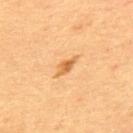Recorded during total-body skin imaging; not selected for excision or biopsy.
The total-body-photography lesion software estimated a lesion area of about 3.5 mm² and a shape-asymmetry score of about 0.25 (0 = symmetric). The software also gave a lesion color around L≈58 a*≈22 b*≈42 in CIELAB, roughly 10 lightness units darker than nearby skin, and a normalized border contrast of about 7.
Imaged with cross-polarized lighting.
A male patient, roughly 60 years of age.
A 15 mm crop from a total-body photograph taken for skin-cancer surveillance.
The lesion is on the upper back.
Approximately 3.5 mm at its widest.